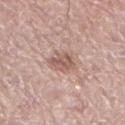Case summary:
* workup: total-body-photography surveillance lesion; no biopsy
* illumination: white-light
* site: the left lower leg
* image source: total-body-photography crop, ~15 mm field of view
* patient: male, aged 58 to 62
* size: ~3.5 mm (longest diameter)